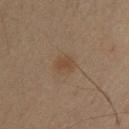Clinical impression:
The lesion was tiled from a total-body skin photograph and was not biopsied.
Background:
This image is a 15 mm lesion crop taken from a total-body photograph. A male subject, roughly 35 years of age. On the upper back. An algorithmic analysis of the crop reported a lesion area of about 6 mm² and an outline eccentricity of about 0.25 (0 = round, 1 = elongated). The software also gave a border-irregularity index near 1/10 and peripheral color asymmetry of about 0.5. The analysis additionally found a detector confidence of about 100 out of 100 that the crop contains a lesion. Captured under cross-polarized illumination.